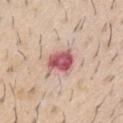The lesion was tiled from a total-body skin photograph and was not biopsied. This image is a 15 mm lesion crop taken from a total-body photograph. The patient is a male in their 60s. The total-body-photography lesion software estimated a border-irregularity rating of about 2.5/10 and internal color variation of about 5.5 on a 0–10 scale. Captured under white-light illumination. Measured at roughly 3.5 mm in maximum diameter. On the arm.